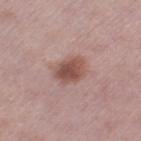The lesion was photographed on a routine skin check and not biopsied; there is no pathology result. A female patient roughly 40 years of age. Automated image analysis of the tile measured a footprint of about 8 mm² and a shape eccentricity near 0.7. And it measured border irregularity of about 2 on a 0–10 scale, a within-lesion color-variation index near 4/10, and a peripheral color-asymmetry measure near 1.5. The analysis additionally found an automated nevus-likeness rating near 90 out of 100 and a detector confidence of about 100 out of 100 that the crop contains a lesion. Cropped from a total-body skin-imaging series; the visible field is about 15 mm. Measured at roughly 3.5 mm in maximum diameter. The lesion is located on the leg. The tile uses white-light illumination.